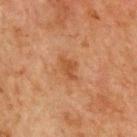Clinical impression:
Captured during whole-body skin photography for melanoma surveillance; the lesion was not biopsied.
Clinical summary:
Cropped from a total-body skin-imaging series; the visible field is about 15 mm. On the chest. Imaged with cross-polarized lighting. The lesion's longest dimension is about 3 mm. The patient is a male about 65 years old.